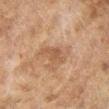  site: leg
  patient:
    sex: female
    age_approx: 60
  image:
    source: total-body photography crop
    field_of_view_mm: 15
  lighting: cross-polarized